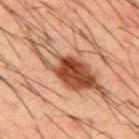Impression:
No biopsy was performed on this lesion — it was imaged during a full skin examination and was not determined to be concerning.
Acquisition and patient details:
A male subject, aged 48–52. Measured at roughly 11 mm in maximum diameter. Cropped from a whole-body photographic skin survey; the tile spans about 15 mm. The lesion is on the mid back.